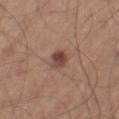Clinical impression:
No biopsy was performed on this lesion — it was imaged during a full skin examination and was not determined to be concerning.
Image and clinical context:
This is a white-light tile. From the right thigh. A roughly 15 mm field-of-view crop from a total-body skin photograph. An algorithmic analysis of the crop reported an average lesion color of about L≈44 a*≈19 b*≈24 (CIELAB), roughly 12 lightness units darker than nearby skin, and a normalized border contrast of about 9. Longest diameter approximately 2.5 mm. The patient is a male about 65 years old.